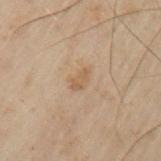- notes · total-body-photography surveillance lesion; no biopsy